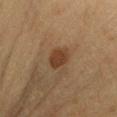The lesion was tiled from a total-body skin photograph and was not biopsied. This image is a 15 mm lesion crop taken from a total-body photograph. On the right upper arm. A female subject about 50 years old.